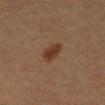notes — imaged on a skin check; not biopsied
image source — total-body-photography crop, ~15 mm field of view
tile lighting — cross-polarized
location — the abdomen
subject — male, approximately 50 years of age